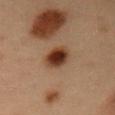follow-up = catalogued during a skin exam; not biopsied | site = the abdomen | diameter = about 3.5 mm | subject = male, about 55 years old | lighting = cross-polarized illumination | acquisition = ~15 mm crop, total-body skin-cancer survey.